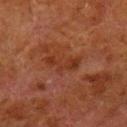A close-up tile cropped from a whole-body skin photograph, about 15 mm across. The patient is a male in their 80s. On the leg. Longest diameter approximately 4 mm. Automated tile analysis of the lesion measured a mean CIELAB color near L≈27 a*≈22 b*≈27 and roughly 6 lightness units darker than nearby skin. The analysis additionally found a border-irregularity rating of about 8/10, a within-lesion color-variation index near 1.5/10, and a peripheral color-asymmetry measure near 0.5. This is a cross-polarized tile.